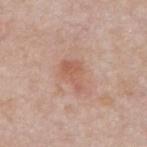<case>
  <biopsy_status>not biopsied; imaged during a skin examination</biopsy_status>
  <automated_metrics>
    <area_mm2_approx>6.5</area_mm2_approx>
    <eccentricity>0.8</eccentricity>
    <shape_asymmetry>0.4</shape_asymmetry>
    <cielab_L>58</cielab_L>
    <cielab_a>22</cielab_a>
    <cielab_b>29</cielab_b>
    <vs_skin_darker_L>8.0</vs_skin_darker_L>
    <border_irregularity_0_10>4.0</border_irregularity_0_10>
    <color_variation_0_10>2.5</color_variation_0_10>
    <peripheral_color_asymmetry>1.0</peripheral_color_asymmetry>
  </automated_metrics>
  <lighting>white-light</lighting>
  <patient>
    <sex>male</sex>
    <age_approx>75</age_approx>
  </patient>
  <lesion_size>
    <long_diameter_mm_approx>3.5</long_diameter_mm_approx>
  </lesion_size>
  <site>back</site>
  <image>
    <source>total-body photography crop</source>
    <field_of_view_mm>15</field_of_view_mm>
  </image>
</case>